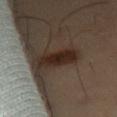Imaged during a routine full-body skin examination; the lesion was not biopsied and no histopathology is available.
This is a cross-polarized tile.
On the left thigh.
A roughly 15 mm field-of-view crop from a total-body skin photograph.
Automated tile analysis of the lesion measured an area of roughly 10 mm² and a shape eccentricity near 0.8.
About 4.5 mm across.
A male subject aged 48–52.